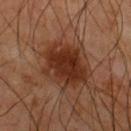Case summary:
• follow-up — imaged on a skin check; not biopsied
• illumination — cross-polarized illumination
• size — about 5.5 mm
• imaging modality — total-body-photography crop, ~15 mm field of view
• subject — male, aged 63–67
• location — the chest